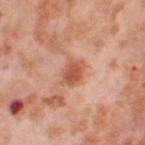{"image": {"source": "total-body photography crop", "field_of_view_mm": 15}, "lighting": "cross-polarized", "site": "right thigh", "lesion_size": {"long_diameter_mm_approx": 3.0}, "patient": {"sex": "female", "age_approx": 55}}